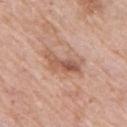Case summary:
* workup: no biopsy performed (imaged during a skin exam)